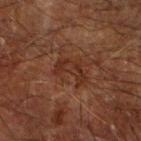Assessment:
Imaged during a routine full-body skin examination; the lesion was not biopsied and no histopathology is available.
Acquisition and patient details:
The lesion's longest dimension is about 3.5 mm. Captured under cross-polarized illumination. A male patient, aged approximately 65. The lesion is on the right forearm. An algorithmic analysis of the crop reported a border-irregularity rating of about 6.5/10, a color-variation rating of about 2.5/10, and peripheral color asymmetry of about 0.5. It also reported an automated nevus-likeness rating near 0 out of 100 and a detector confidence of about 100 out of 100 that the crop contains a lesion. A lesion tile, about 15 mm wide, cut from a 3D total-body photograph.